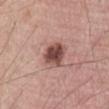Notes:
- notes · no biopsy performed (imaged during a skin exam)
- automated lesion analysis · an area of roughly 8.5 mm², a shape eccentricity near 0.4, and a shape-asymmetry score of about 0.2 (0 = symmetric); a border-irregularity rating of about 1.5/10, a color-variation rating of about 4.5/10, and radial color variation of about 1.5
- site · the abdomen
- tile lighting · white-light
- subject · male, about 70 years old
- acquisition · ~15 mm crop, total-body skin-cancer survey